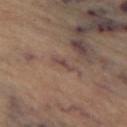Impression:
This lesion was catalogued during total-body skin photography and was not selected for biopsy.
Acquisition and patient details:
From the left thigh. Measured at roughly 3 mm in maximum diameter. A subject approximately 60 years of age. Cropped from a whole-body photographic skin survey; the tile spans about 15 mm.